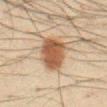Case summary:
– follow-up — imaged on a skin check; not biopsied
– body site — the abdomen
– image source — 15 mm crop, total-body photography
– TBP lesion metrics — an area of roughly 14 mm², a shape eccentricity near 0.55, and a shape-asymmetry score of about 0.2 (0 = symmetric); a within-lesion color-variation index near 3.5/10 and peripheral color asymmetry of about 1; a nevus-likeness score of about 100/100 and lesion-presence confidence of about 100/100
– lesion size — ≈4.5 mm
– subject — male, aged 58–62
– illumination — cross-polarized illumination